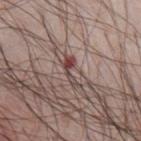Q: Was a biopsy performed?
A: no biopsy performed (imaged during a skin exam)
Q: What is the lesion's diameter?
A: ≈3.5 mm
Q: Automated lesion metrics?
A: a footprint of about 3.5 mm² and two-axis asymmetry of about 0.6
Q: What are the patient's age and sex?
A: male, about 60 years old
Q: What is the imaging modality?
A: 15 mm crop, total-body photography
Q: What is the anatomic site?
A: the chest